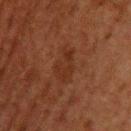workup = total-body-photography surveillance lesion; no biopsy | diameter = about 4.5 mm | subject = male, aged 58 to 62 | image-analysis metrics = a classifier nevus-likeness of about 5/100 | location = the upper back | acquisition = total-body-photography crop, ~15 mm field of view.